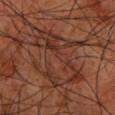This lesion was catalogued during total-body skin photography and was not selected for biopsy.
About 7.5 mm across.
The tile uses cross-polarized illumination.
On the left forearm.
The patient is a male about 60 years old.
Automated image analysis of the tile measured a footprint of about 30 mm², an eccentricity of roughly 0.65, and two-axis asymmetry of about 0.3. The analysis additionally found a mean CIELAB color near L≈33 a*≈22 b*≈27, a lesion–skin lightness drop of about 6, and a normalized border contrast of about 6.
This image is a 15 mm lesion crop taken from a total-body photograph.